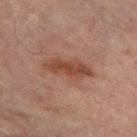| key | value |
|---|---|
| notes | imaged on a skin check; not biopsied |
| patient | female, in their 80s |
| image | total-body-photography crop, ~15 mm field of view |
| anatomic site | the right leg |
| image-analysis metrics | a shape eccentricity near 0.9 and a symmetry-axis asymmetry near 0.25; a lesion color around L≈41 a*≈21 b*≈27 in CIELAB and roughly 9 lightness units darker than nearby skin |
| lighting | cross-polarized |
| lesion diameter | ~5.5 mm (longest diameter) |